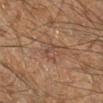Q: Was a biopsy performed?
A: total-body-photography surveillance lesion; no biopsy
Q: How was the tile lit?
A: cross-polarized
Q: What are the patient's age and sex?
A: male, approximately 60 years of age
Q: How large is the lesion?
A: ~2.5 mm (longest diameter)
Q: Where on the body is the lesion?
A: the leg
Q: What is the imaging modality?
A: ~15 mm tile from a whole-body skin photo